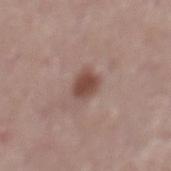<case>
<biopsy_status>not biopsied; imaged during a skin examination</biopsy_status>
<patient>
  <sex>male</sex>
  <age_approx>70</age_approx>
</patient>
<lighting>white-light</lighting>
<automated_metrics>
  <area_mm2_approx>5.5</area_mm2_approx>
  <eccentricity>0.5</eccentricity>
  <shape_asymmetry>0.2</shape_asymmetry>
  <cielab_L>47</cielab_L>
  <cielab_a>20</cielab_a>
  <cielab_b>23</cielab_b>
  <vs_skin_darker_L>12.0</vs_skin_darker_L>
  <vs_skin_contrast_norm>9.0</vs_skin_contrast_norm>
  <border_irregularity_0_10>1.5</border_irregularity_0_10>
  <color_variation_0_10>2.0</color_variation_0_10>
  <peripheral_color_asymmetry>0.5</peripheral_color_asymmetry>
  <nevus_likeness_0_100>95</nevus_likeness_0_100>
  <lesion_detection_confidence_0_100>100</lesion_detection_confidence_0_100>
</automated_metrics>
<image>
  <source>total-body photography crop</source>
  <field_of_view_mm>15</field_of_view_mm>
</image>
<site>back</site>
<lesion_size>
  <long_diameter_mm_approx>3.0</long_diameter_mm_approx>
</lesion_size>
</case>